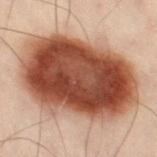Imaged during a routine full-body skin examination; the lesion was not biopsied and no histopathology is available. A region of skin cropped from a whole-body photographic capture, roughly 15 mm wide. The lesion-visualizer software estimated an average lesion color of about L≈39 a*≈21 b*≈27 (CIELAB), a lesion–skin lightness drop of about 19, and a normalized border contrast of about 15. And it measured a nevus-likeness score of about 100/100. The patient is a male aged 48–52. Longest diameter approximately 11.5 mm. Located on the left leg. Imaged with cross-polarized lighting.